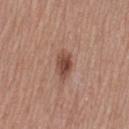Case summary:
- subject: female, aged approximately 55
- site: the left thigh
- diameter: ≈3.5 mm
- TBP lesion metrics: a mean CIELAB color near L≈47 a*≈22 b*≈27, a lesion–skin lightness drop of about 12, and a normalized lesion–skin contrast near 9; border irregularity of about 2 on a 0–10 scale
- illumination: white-light illumination
- image: 15 mm crop, total-body photography